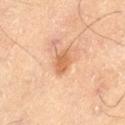workup = catalogued during a skin exam; not biopsied
subject = male, about 70 years old
diameter = about 3 mm
illumination = cross-polarized
site = the left thigh
acquisition = ~15 mm crop, total-body skin-cancer survey